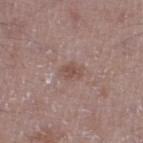Q: Is there a histopathology result?
A: no biopsy performed (imaged during a skin exam)
Q: What is the imaging modality?
A: total-body-photography crop, ~15 mm field of view
Q: What lighting was used for the tile?
A: white-light illumination
Q: What is the anatomic site?
A: the right thigh
Q: Automated lesion metrics?
A: a footprint of about 4 mm² and an outline eccentricity of about 0.75 (0 = round, 1 = elongated); an average lesion color of about L≈50 a*≈18 b*≈23 (CIELAB) and a lesion–skin lightness drop of about 8; a border-irregularity rating of about 2.5/10 and a peripheral color-asymmetry measure near 1; an automated nevus-likeness rating near 0 out of 100 and a detector confidence of about 100 out of 100 that the crop contains a lesion
Q: What is the lesion's diameter?
A: ≈2.5 mm
Q: Patient demographics?
A: male, aged 48 to 52